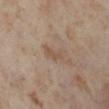Recorded during total-body skin imaging; not selected for excision or biopsy. Imaged with cross-polarized lighting. Longest diameter approximately 3 mm. The lesion is on the left lower leg. Cropped from a total-body skin-imaging series; the visible field is about 15 mm. A female patient aged around 55. The lesion-visualizer software estimated roughly 6 lightness units darker than nearby skin and a lesion-to-skin contrast of about 5.5 (normalized; higher = more distinct). The software also gave a within-lesion color-variation index near 0/10 and peripheral color asymmetry of about 0.